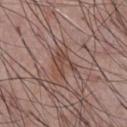| feature | finding |
|---|---|
| follow-up | no biopsy performed (imaged during a skin exam) |
| patient | male, in their mid-50s |
| lesion size | ~3.5 mm (longest diameter) |
| imaging modality | total-body-photography crop, ~15 mm field of view |
| illumination | white-light illumination |
| anatomic site | the chest |